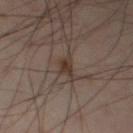Part of a total-body skin-imaging series; this lesion was reviewed on a skin check and was not flagged for biopsy.
Cropped from a whole-body photographic skin survey; the tile spans about 15 mm.
Approximately 2.5 mm at its widest.
The lesion is located on the left thigh.
This is a cross-polarized tile.
A male patient, aged 53–57.